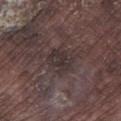Imaged during a routine full-body skin examination; the lesion was not biopsied and no histopathology is available. An algorithmic analysis of the crop reported a footprint of about 7 mm², an outline eccentricity of about 0.65 (0 = round, 1 = elongated), and a symmetry-axis asymmetry near 0.2. The analysis additionally found border irregularity of about 3 on a 0–10 scale, a within-lesion color-variation index near 3/10, and peripheral color asymmetry of about 1. The analysis additionally found lesion-presence confidence of about 75/100. The lesion is on the left lower leg. The recorded lesion diameter is about 3.5 mm. The subject is a male aged approximately 75. A 15 mm close-up extracted from a 3D total-body photography capture. Imaged with white-light lighting.